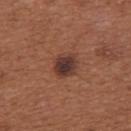The lesion was photographed on a routine skin check and not biopsied; there is no pathology result.
This is a white-light tile.
A region of skin cropped from a whole-body photographic capture, roughly 15 mm wide.
The total-body-photography lesion software estimated an average lesion color of about L≈36 a*≈21 b*≈24 (CIELAB) and roughly 12 lightness units darker than nearby skin. It also reported a border-irregularity rating of about 2/10 and radial color variation of about 1.5. The software also gave a classifier nevus-likeness of about 85/100 and a detector confidence of about 100 out of 100 that the crop contains a lesion.
A female subject, approximately 65 years of age.
The lesion is located on the back.
The recorded lesion diameter is about 3 mm.